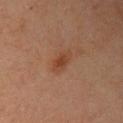Imaged during a routine full-body skin examination; the lesion was not biopsied and no histopathology is available.
Cropped from a total-body skin-imaging series; the visible field is about 15 mm.
This is a cross-polarized tile.
A female subject, in their mid- to late 60s.
The lesion is on the right upper arm.
About 3 mm across.